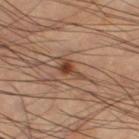{"biopsy_status": "not biopsied; imaged during a skin examination", "image": {"source": "total-body photography crop", "field_of_view_mm": 15}, "site": "right thigh", "lighting": "cross-polarized", "lesion_size": {"long_diameter_mm_approx": 3.0}, "patient": {"sex": "male", "age_approx": 60}, "automated_metrics": {"border_irregularity_0_10": 5.5, "color_variation_0_10": 2.5, "nevus_likeness_0_100": 95, "lesion_detection_confidence_0_100": 100}}